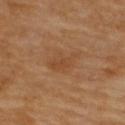workup=total-body-photography surveillance lesion; no biopsy | lighting=cross-polarized illumination | imaging modality=total-body-photography crop, ~15 mm field of view | location=the upper back | subject=female, aged 58–62 | diameter=≈4 mm.